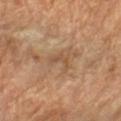Assessment: The lesion was tiled from a total-body skin photograph and was not biopsied. Clinical summary: An algorithmic analysis of the crop reported an area of roughly 3.5 mm² and an outline eccentricity of about 0.85 (0 = round, 1 = elongated). It also reported a mean CIELAB color near L≈49 a*≈17 b*≈32, roughly 7 lightness units darker than nearby skin, and a lesion-to-skin contrast of about 5 (normalized; higher = more distinct). This image is a 15 mm lesion crop taken from a total-body photograph. From the left forearm. Approximately 3 mm at its widest. This is a cross-polarized tile. A female patient approximately 70 years of age.